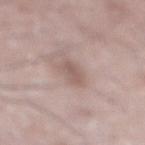The lesion was tiled from a total-body skin photograph and was not biopsied.
The subject is a male aged approximately 70.
The lesion is on the left leg.
This is a white-light tile.
A close-up tile cropped from a whole-body skin photograph, about 15 mm across.
Automated image analysis of the tile measured a mean CIELAB color near L≈57 a*≈16 b*≈22, roughly 8 lightness units darker than nearby skin, and a normalized border contrast of about 6. The software also gave a nevus-likeness score of about 5/100 and a lesion-detection confidence of about 100/100.